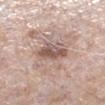location = the right lower leg; image source = ~15 mm tile from a whole-body skin photo; subject = male, aged approximately 75; lighting = white-light illumination; lesion diameter = ~4 mm (longest diameter).